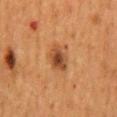follow-up: no biopsy performed (imaged during a skin exam) | image: ~15 mm tile from a whole-body skin photo | patient: female, aged 48 to 52 | diameter: ≈3.5 mm | tile lighting: cross-polarized illumination | anatomic site: the mid back.